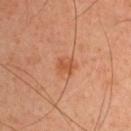Imaged during a routine full-body skin examination; the lesion was not biopsied and no histopathology is available. The lesion's longest dimension is about 2.5 mm. A male subject aged 43–47. On the left upper arm. Automated tile analysis of the lesion measured an area of roughly 4.5 mm² and a shape eccentricity near 0.4. Cropped from a total-body skin-imaging series; the visible field is about 15 mm.